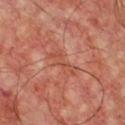Assessment:
Imaged during a routine full-body skin examination; the lesion was not biopsied and no histopathology is available.
Image and clinical context:
A 15 mm close-up extracted from a 3D total-body photography capture. Automated image analysis of the tile measured an automated nevus-likeness rating near 0 out of 100 and a lesion-detection confidence of about 90/100. Located on the chest. Imaged with cross-polarized lighting. Approximately 4 mm at its widest. The patient is a male approximately 55 years of age.